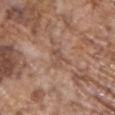No biopsy was performed on this lesion — it was imaged during a full skin examination and was not determined to be concerning. Longest diameter approximately 2.5 mm. Cropped from a total-body skin-imaging series; the visible field is about 15 mm. A male patient aged 68–72. The lesion is on the chest.